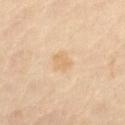Part of a total-body skin-imaging series; this lesion was reviewed on a skin check and was not flagged for biopsy.
Cropped from a whole-body photographic skin survey; the tile spans about 15 mm.
A male patient aged around 65.
Measured at roughly 2.5 mm in maximum diameter.
On the abdomen.